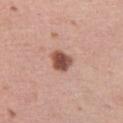Imaged during a routine full-body skin examination; the lesion was not biopsied and no histopathology is available. The patient is a female about 40 years old. From the left thigh. About 3 mm across. A 15 mm crop from a total-body photograph taken for skin-cancer surveillance.